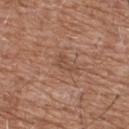The patient is a male aged 58 to 62.
This image is a 15 mm lesion crop taken from a total-body photograph.
Located on the chest.
The recorded lesion diameter is about 2.5 mm.
The total-body-photography lesion software estimated a lesion area of about 2.5 mm² and an outline eccentricity of about 0.9 (0 = round, 1 = elongated). The analysis additionally found roughly 7 lightness units darker than nearby skin and a normalized border contrast of about 5. The software also gave a border-irregularity index near 6/10, a color-variation rating of about 0/10, and radial color variation of about 0. It also reported an automated nevus-likeness rating near 0 out of 100 and a lesion-detection confidence of about 100/100.
This is a white-light tile.